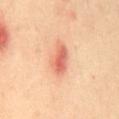This lesion was catalogued during total-body skin photography and was not selected for biopsy. Captured under cross-polarized illumination. From the mid back. The patient is a female aged 38 to 42. Longest diameter approximately 4.5 mm. A roughly 15 mm field-of-view crop from a total-body skin photograph.